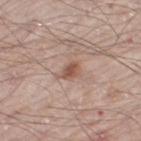Q: Was a biopsy performed?
A: no biopsy performed (imaged during a skin exam)
Q: What kind of image is this?
A: total-body-photography crop, ~15 mm field of view
Q: What are the patient's age and sex?
A: male, aged 58–62
Q: Where on the body is the lesion?
A: the right thigh
Q: What did automated image analysis measure?
A: a footprint of about 3.5 mm², an eccentricity of roughly 0.75, and two-axis asymmetry of about 0.2; an average lesion color of about L≈53 a*≈20 b*≈27 (CIELAB), a lesion–skin lightness drop of about 10, and a normalized border contrast of about 7.5; internal color variation of about 2.5 on a 0–10 scale and peripheral color asymmetry of about 1
Q: Illumination type?
A: white-light illumination
Q: What is the lesion's diameter?
A: ≈2.5 mm